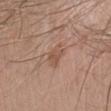On the left forearm.
This is a white-light tile.
A roughly 15 mm field-of-view crop from a total-body skin photograph.
A male patient, about 65 years old.
Measured at roughly 3 mm in maximum diameter.